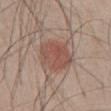<lesion>
  <biopsy_status>not biopsied; imaged during a skin examination</biopsy_status>
  <site>abdomen</site>
  <image>
    <source>total-body photography crop</source>
    <field_of_view_mm>15</field_of_view_mm>
  </image>
  <patient>
    <sex>male</sex>
    <age_approx>50</age_approx>
  </patient>
  <lighting>white-light</lighting>
  <automated_metrics>
    <area_mm2_approx>14.0</area_mm2_approx>
    <shape_asymmetry>0.2</shape_asymmetry>
    <cielab_L>51</cielab_L>
    <cielab_a>21</cielab_a>
    <cielab_b>25</cielab_b>
    <vs_skin_darker_L>9.0</vs_skin_darker_L>
    <vs_skin_contrast_norm>6.5</vs_skin_contrast_norm>
    <border_irregularity_0_10>2.0</border_irregularity_0_10>
    <color_variation_0_10>3.5</color_variation_0_10>
  </automated_metrics>
  <lesion_size>
    <long_diameter_mm_approx>4.5</long_diameter_mm_approx>
  </lesion_size>
</lesion>